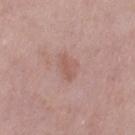biopsy status: no biopsy performed (imaged during a skin exam); subject: female, aged approximately 40; image: total-body-photography crop, ~15 mm field of view; TBP lesion metrics: a detector confidence of about 100 out of 100 that the crop contains a lesion; illumination: white-light illumination; anatomic site: the left thigh.